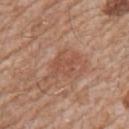workup = total-body-photography surveillance lesion; no biopsy | body site = the right upper arm | patient = male, approximately 60 years of age | image source = total-body-photography crop, ~15 mm field of view.